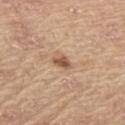image source: total-body-photography crop, ~15 mm field of view
tile lighting: white-light illumination
body site: the upper back
patient: male, aged 63 to 67
automated metrics: an area of roughly 3.5 mm², a shape eccentricity near 0.8, and a shape-asymmetry score of about 0.3 (0 = symmetric); a border-irregularity index near 2.5/10, internal color variation of about 2.5 on a 0–10 scale, and radial color variation of about 1; an automated nevus-likeness rating near 80 out of 100